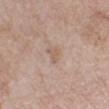Clinical impression: The lesion was photographed on a routine skin check and not biopsied; there is no pathology result. Image and clinical context: Approximately 2.5 mm at its widest. Located on the left lower leg. Cropped from a whole-body photographic skin survey; the tile spans about 15 mm. A female subject approximately 70 years of age. Automated tile analysis of the lesion measured a classifier nevus-likeness of about 0/100.